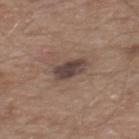Imaged during a routine full-body skin examination; the lesion was not biopsied and no histopathology is available. The lesion is located on the mid back. This is a white-light tile. Longest diameter approximately 3.5 mm. Automated image analysis of the tile measured an eccentricity of roughly 0.75 and two-axis asymmetry of about 0.2. It also reported an average lesion color of about L≈41 a*≈15 b*≈19 (CIELAB). The analysis additionally found a nevus-likeness score of about 15/100 and a detector confidence of about 100 out of 100 that the crop contains a lesion. This image is a 15 mm lesion crop taken from a total-body photograph. A male patient roughly 65 years of age.